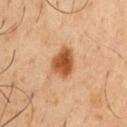Case summary:
- biopsy status · catalogued during a skin exam; not biopsied
- TBP lesion metrics · an average lesion color of about L≈54 a*≈25 b*≈40 (CIELAB), a lesion–skin lightness drop of about 15, and a normalized border contrast of about 10.5
- location · the chest
- patient · male, about 50 years old
- illumination · cross-polarized
- imaging modality · 15 mm crop, total-body photography